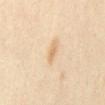Captured during whole-body skin photography for melanoma surveillance; the lesion was not biopsied. Captured under cross-polarized illumination. The recorded lesion diameter is about 2.5 mm. A 15 mm close-up extracted from a 3D total-body photography capture. A female subject, roughly 35 years of age. Located on the mid back.